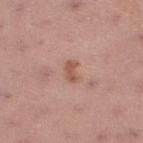| field | value |
|---|---|
| anatomic site | the left lower leg |
| image | ~15 mm tile from a whole-body skin photo |
| lesion size | ~2.5 mm (longest diameter) |
| subject | female, approximately 55 years of age |
| TBP lesion metrics | a footprint of about 3.5 mm², a shape eccentricity near 0.8, and two-axis asymmetry of about 0.3; a border-irregularity index near 2.5/10, internal color variation of about 1.5 on a 0–10 scale, and radial color variation of about 0.5; an automated nevus-likeness rating near 5 out of 100 |
| illumination | white-light |